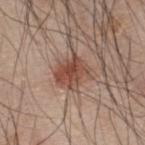follow-up: imaged on a skin check; not biopsied | size: ~4 mm (longest diameter) | tile lighting: white-light | subject: male, aged approximately 45 | anatomic site: the back | image: ~15 mm crop, total-body skin-cancer survey | TBP lesion metrics: an area of roughly 11 mm², an outline eccentricity of about 0.5 (0 = round, 1 = elongated), and a shape-asymmetry score of about 0.25 (0 = symmetric); an automated nevus-likeness rating near 95 out of 100 and a lesion-detection confidence of about 100/100.